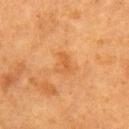<case>
  <site>right upper arm</site>
  <lesion_size>
    <long_diameter_mm_approx>3.0</long_diameter_mm_approx>
  </lesion_size>
  <lighting>cross-polarized</lighting>
  <image>
    <source>total-body photography crop</source>
    <field_of_view_mm>15</field_of_view_mm>
  </image>
  <patient>
    <sex>female</sex>
    <age_approx>55</age_approx>
  </patient>
</case>